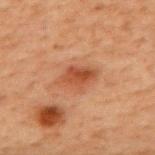Recorded during total-body skin imaging; not selected for excision or biopsy.
A male subject, aged around 70.
A region of skin cropped from a whole-body photographic capture, roughly 15 mm wide.
Captured under cross-polarized illumination.
Longest diameter approximately 4 mm.
The lesion is on the upper back.